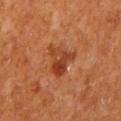Part of a total-body skin-imaging series; this lesion was reviewed on a skin check and was not flagged for biopsy.
Located on the mid back.
A lesion tile, about 15 mm wide, cut from a 3D total-body photograph.
This is a cross-polarized tile.
The patient is a male aged 63 to 67.